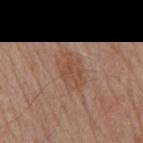Impression: The lesion was tiled from a total-body skin photograph and was not biopsied. Background: A male subject aged approximately 80. This is a white-light tile. A region of skin cropped from a whole-body photographic capture, roughly 15 mm wide. The lesion's longest dimension is about 4.5 mm. Automated image analysis of the tile measured an area of roughly 8.5 mm² and a shape eccentricity near 0.8. It also reported border irregularity of about 4 on a 0–10 scale and a color-variation rating of about 2.5/10. The lesion is on the mid back.